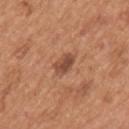<tbp_lesion>
<biopsy_status>not biopsied; imaged during a skin examination</biopsy_status>
<site>arm</site>
<lighting>white-light</lighting>
<lesion_size>
  <long_diameter_mm_approx>3.0</long_diameter_mm_approx>
</lesion_size>
<patient>
  <sex>male</sex>
  <age_approx>65</age_approx>
</patient>
<image>
  <source>total-body photography crop</source>
  <field_of_view_mm>15</field_of_view_mm>
</image>
</tbp_lesion>